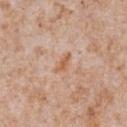Clinical impression: This lesion was catalogued during total-body skin photography and was not selected for biopsy. Context: Captured under white-light illumination. A male subject aged 63 to 67. A 15 mm close-up tile from a total-body photography series done for melanoma screening. Located on the front of the torso. The lesion's longest dimension is about 3 mm.